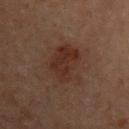The lesion was tiled from a total-body skin photograph and was not biopsied. A roughly 15 mm field-of-view crop from a total-body skin photograph. This is a cross-polarized tile. The total-body-photography lesion software estimated a lesion area of about 16 mm² and an eccentricity of roughly 0.75. And it measured an average lesion color of about L≈22 a*≈15 b*≈19 (CIELAB) and about 5 CIELAB-L* units darker than the surrounding skin. It also reported a color-variation rating of about 2.5/10 and peripheral color asymmetry of about 1. Located on the back. The patient is a male roughly 70 years of age.